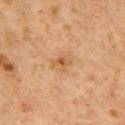{"biopsy_status": "not biopsied; imaged during a skin examination", "lighting": "cross-polarized", "image": {"source": "total-body photography crop", "field_of_view_mm": 15}, "patient": {"sex": "male", "age_approx": 60}, "site": "chest"}